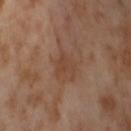The lesion was tiled from a total-body skin photograph and was not biopsied.
The lesion is located on the right thigh.
The total-body-photography lesion software estimated a footprint of about 5 mm², an outline eccentricity of about 0.65 (0 = round, 1 = elongated), and a symmetry-axis asymmetry near 0.4. The software also gave a lesion color around L≈43 a*≈19 b*≈29 in CIELAB, roughly 6 lightness units darker than nearby skin, and a normalized lesion–skin contrast near 5.
A female subject, aged around 55.
A 15 mm close-up tile from a total-body photography series done for melanoma screening.
Longest diameter approximately 3 mm.
The tile uses cross-polarized illumination.